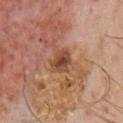Clinical impression: This lesion was catalogued during total-body skin photography and was not selected for biopsy. Background: Cropped from a total-body skin-imaging series; the visible field is about 15 mm. From the chest. This is a cross-polarized tile. A male subject aged approximately 60.